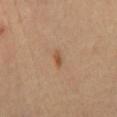Q: Is there a histopathology result?
A: no biopsy performed (imaged during a skin exam)
Q: How large is the lesion?
A: ≈2 mm
Q: What are the patient's age and sex?
A: male, aged 58 to 62
Q: Lesion location?
A: the chest
Q: What is the imaging modality?
A: total-body-photography crop, ~15 mm field of view